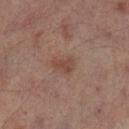<case>
  <biopsy_status>not biopsied; imaged during a skin examination</biopsy_status>
  <image>
    <source>total-body photography crop</source>
    <field_of_view_mm>15</field_of_view_mm>
  </image>
  <site>left lower leg</site>
  <patient>
    <sex>male</sex>
    <age_approx>65</age_approx>
  </patient>
</case>